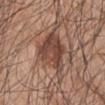Case summary:
- biopsy status: total-body-photography surveillance lesion; no biopsy
- lesion size: about 6 mm
- tile lighting: white-light
- subject: male, in their mid- to late 40s
- automated metrics: an area of roughly 17 mm²; a within-lesion color-variation index near 5.5/10; a nevus-likeness score of about 75/100
- acquisition: ~15 mm crop, total-body skin-cancer survey
- body site: the left upper arm